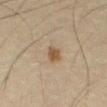The lesion was photographed on a routine skin check and not biopsied; there is no pathology result.